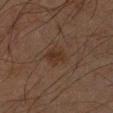Clinical summary:
On the left forearm. The recorded lesion diameter is about 2.5 mm. A male subject, aged 63–67. A close-up tile cropped from a whole-body skin photograph, about 15 mm across. Captured under cross-polarized illumination.